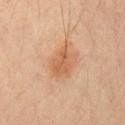<record>
  <biopsy_status>not biopsied; imaged during a skin examination</biopsy_status>
  <site>chest</site>
  <patient>
    <sex>male</sex>
    <age_approx>35</age_approx>
  </patient>
  <image>
    <source>total-body photography crop</source>
    <field_of_view_mm>15</field_of_view_mm>
  </image>
</record>